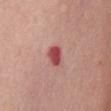Case summary:
* illumination: white-light
* image: ~15 mm crop, total-body skin-cancer survey
* diameter: ≈2.5 mm
* subject: female, roughly 65 years of age
* body site: the chest